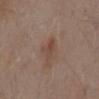Assessment: No biopsy was performed on this lesion — it was imaged during a full skin examination and was not determined to be concerning. Background: On the mid back. A 15 mm close-up extracted from a 3D total-body photography capture. The tile uses white-light illumination. A male patient, aged approximately 55.